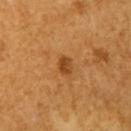The lesion was tiled from a total-body skin photograph and was not biopsied.
A female subject, in their mid-50s.
Cropped from a whole-body photographic skin survey; the tile spans about 15 mm.
The lesion is on the left upper arm.